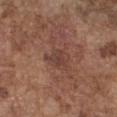Impression: This lesion was catalogued during total-body skin photography and was not selected for biopsy. Clinical summary: Located on the chest. Imaged with white-light lighting. About 3 mm across. Cropped from a total-body skin-imaging series; the visible field is about 15 mm. A male patient aged 73 to 77.